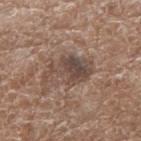Recorded during total-body skin imaging; not selected for excision or biopsy. A female patient roughly 75 years of age. A 15 mm close-up tile from a total-body photography series done for melanoma screening. This is a white-light tile. Located on the arm.